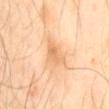Findings:
– notes — total-body-photography surveillance lesion; no biopsy
– lesion diameter — about 3.5 mm
– tile lighting — cross-polarized
– site — the mid back
– image source — total-body-photography crop, ~15 mm field of view
– subject — male, in their mid-60s